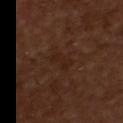Assessment: This lesion was catalogued during total-body skin photography and was not selected for biopsy. Acquisition and patient details: Located on the chest. Automated tile analysis of the lesion measured an average lesion color of about L≈21 a*≈17 b*≈23 (CIELAB), roughly 4 lightness units darker than nearby skin, and a lesion-to-skin contrast of about 5 (normalized; higher = more distinct). The patient is a male aged 48 to 52. About 3 mm across. A lesion tile, about 15 mm wide, cut from a 3D total-body photograph. Imaged with cross-polarized lighting.